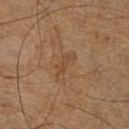Part of a total-body skin-imaging series; this lesion was reviewed on a skin check and was not flagged for biopsy. Located on the right lower leg. Imaged with cross-polarized lighting. Approximately 2.5 mm at its widest. The subject is a male aged 58 to 62. The total-body-photography lesion software estimated a lesion color around L≈43 a*≈18 b*≈31 in CIELAB, a lesion–skin lightness drop of about 6, and a normalized lesion–skin contrast near 5. It also reported a border-irregularity index near 3/10, internal color variation of about 0 on a 0–10 scale, and peripheral color asymmetry of about 0. And it measured a nevus-likeness score of about 0/100 and lesion-presence confidence of about 100/100. A 15 mm close-up extracted from a 3D total-body photography capture.